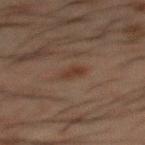Notes:
• biopsy status · imaged on a skin check; not biopsied
• illumination · cross-polarized illumination
• body site · the mid back
• subject · male, approximately 50 years of age
• acquisition · total-body-photography crop, ~15 mm field of view
• diameter · about 2.5 mm